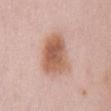workup = total-body-photography surveillance lesion; no biopsy
image-analysis metrics = an average lesion color of about L≈59 a*≈22 b*≈30 (CIELAB) and roughly 13 lightness units darker than nearby skin; border irregularity of about 2.5 on a 0–10 scale, a within-lesion color-variation index near 5/10, and radial color variation of about 1.5
image = ~15 mm crop, total-body skin-cancer survey
subject = female, aged approximately 65
illumination = white-light illumination
lesion size = ≈5.5 mm
anatomic site = the mid back